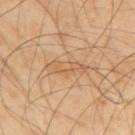Assessment: Part of a total-body skin-imaging series; this lesion was reviewed on a skin check and was not flagged for biopsy. Acquisition and patient details: Approximately 4.5 mm at its widest. The lesion is located on the upper back. Imaged with cross-polarized lighting. The patient is a male aged approximately 40. A 15 mm crop from a total-body photograph taken for skin-cancer surveillance.